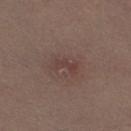Assessment:
The lesion was photographed on a routine skin check and not biopsied; there is no pathology result.
Background:
A female subject about 30 years old. A lesion tile, about 15 mm wide, cut from a 3D total-body photograph. Measured at roughly 3 mm in maximum diameter. The tile uses white-light illumination. The lesion-visualizer software estimated a footprint of about 3.5 mm², a shape eccentricity near 0.9, and a symmetry-axis asymmetry near 0.35. The analysis additionally found a color-variation rating of about 0/10 and a peripheral color-asymmetry measure near 0. The software also gave a classifier nevus-likeness of about 0/100. Located on the leg.